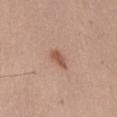Assessment: Part of a total-body skin-imaging series; this lesion was reviewed on a skin check and was not flagged for biopsy. Context: This is a white-light tile. The total-body-photography lesion software estimated a mean CIELAB color near L≈54 a*≈22 b*≈30 and a normalized lesion–skin contrast near 7.5. The analysis additionally found a classifier nevus-likeness of about 85/100 and lesion-presence confidence of about 100/100. A male patient, in their mid-50s. Located on the abdomen. Cropped from a whole-body photographic skin survey; the tile spans about 15 mm.